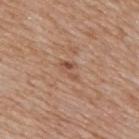image:
  source: total-body photography crop
  field_of_view_mm: 15
lesion_size:
  long_diameter_mm_approx: 2.5
automated_metrics:
  area_mm2_approx: 2.5
  shape_asymmetry: 0.45
  border_irregularity_0_10: 6.0
  color_variation_0_10: 0.0
  peripheral_color_asymmetry: 0.0
  lesion_detection_confidence_0_100: 100
lighting: white-light
site: mid back
patient:
  sex: male
  age_approx: 60